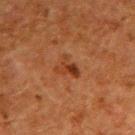No biopsy was performed on this lesion — it was imaged during a full skin examination and was not determined to be concerning. Automated image analysis of the tile measured a lesion area of about 5 mm² and two-axis asymmetry of about 0.5. It also reported a mean CIELAB color near L≈30 a*≈22 b*≈30, a lesion–skin lightness drop of about 7, and a lesion-to-skin contrast of about 7 (normalized; higher = more distinct). The software also gave a border-irregularity index near 5.5/10, internal color variation of about 5 on a 0–10 scale, and radial color variation of about 1.5. And it measured a nevus-likeness score of about 5/100 and lesion-presence confidence of about 100/100. A male patient about 60 years old. A 15 mm close-up extracted from a 3D total-body photography capture. Located on the arm. The recorded lesion diameter is about 3.5 mm.